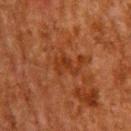{"biopsy_status": "not biopsied; imaged during a skin examination", "site": "upper back", "patient": {"sex": "male", "age_approx": 60}, "image": {"source": "total-body photography crop", "field_of_view_mm": 15}}